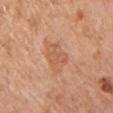Automated image analysis of the tile measured a footprint of about 9.5 mm², a shape eccentricity near 0.65, and two-axis asymmetry of about 0.3. The analysis additionally found a lesion color around L≈59 a*≈23 b*≈35 in CIELAB, roughly 7 lightness units darker than nearby skin, and a lesion-to-skin contrast of about 5 (normalized; higher = more distinct). The software also gave a border-irregularity index near 3/10, a color-variation rating of about 2.5/10, and peripheral color asymmetry of about 1. And it measured a nevus-likeness score of about 0/100.
Located on the chest.
A male subject in their 60s.
Longest diameter approximately 4 mm.
A 15 mm crop from a total-body photograph taken for skin-cancer surveillance.
This is a white-light tile.